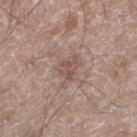Captured during whole-body skin photography for melanoma surveillance; the lesion was not biopsied. The tile uses white-light illumination. The patient is a male aged 58 to 62. From the right lower leg. Cropped from a whole-body photographic skin survey; the tile spans about 15 mm. Automated tile analysis of the lesion measured a lesion color around L≈51 a*≈17 b*≈23 in CIELAB and a lesion-to-skin contrast of about 5.5 (normalized; higher = more distinct). The analysis additionally found border irregularity of about 5.5 on a 0–10 scale and a peripheral color-asymmetry measure near 0.5. And it measured an automated nevus-likeness rating near 0 out of 100.